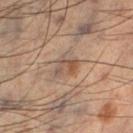Impression: Captured during whole-body skin photography for melanoma surveillance; the lesion was not biopsied. Context: Measured at roughly 3 mm in maximum diameter. A region of skin cropped from a whole-body photographic capture, roughly 15 mm wide. A male patient, aged approximately 50. On the left lower leg. Imaged with cross-polarized lighting.